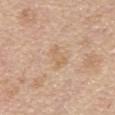<tbp_lesion>
<biopsy_status>not biopsied; imaged during a skin examination</biopsy_status>
<patient>
  <sex>male</sex>
  <age_approx>70</age_approx>
</patient>
<site>mid back</site>
<automated_metrics>
  <vs_skin_darker_L>6.0</vs_skin_darker_L>
  <vs_skin_contrast_norm>4.5</vs_skin_contrast_norm>
</automated_metrics>
<lighting>white-light</lighting>
<image>
  <source>total-body photography crop</source>
  <field_of_view_mm>15</field_of_view_mm>
</image>
<lesion_size>
  <long_diameter_mm_approx>3.0</long_diameter_mm_approx>
</lesion_size>
</tbp_lesion>